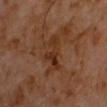{"biopsy_status": "not biopsied; imaged during a skin examination", "image": {"source": "total-body photography crop", "field_of_view_mm": 15}, "site": "chest", "patient": {"sex": "male", "age_approx": 60}}